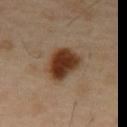No biopsy was performed on this lesion — it was imaged during a full skin examination and was not determined to be concerning. From the mid back. Cropped from a total-body skin-imaging series; the visible field is about 15 mm. The lesion's longest dimension is about 4.5 mm. A male patient aged 43–47.